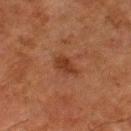Recorded during total-body skin imaging; not selected for excision or biopsy. This is a cross-polarized tile. A male patient, aged 78–82. This image is a 15 mm lesion crop taken from a total-body photograph. The lesion is located on the left thigh.